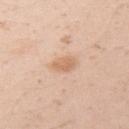Q: Was a biopsy performed?
A: catalogued during a skin exam; not biopsied
Q: How was the tile lit?
A: white-light
Q: Patient demographics?
A: female, in their 20s
Q: What is the anatomic site?
A: the left upper arm
Q: What kind of image is this?
A: 15 mm crop, total-body photography